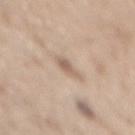This lesion was catalogued during total-body skin photography and was not selected for biopsy.
The lesion is located on the mid back.
A lesion tile, about 15 mm wide, cut from a 3D total-body photograph.
A male subject, aged 68 to 72.
Measured at roughly 2.5 mm in maximum diameter.